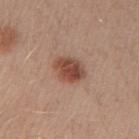Q: Was a biopsy performed?
A: imaged on a skin check; not biopsied
Q: How was the tile lit?
A: white-light
Q: Who is the patient?
A: male, aged 28–32
Q: How was this image acquired?
A: ~15 mm crop, total-body skin-cancer survey
Q: Where on the body is the lesion?
A: the right upper arm
Q: How large is the lesion?
A: about 4 mm